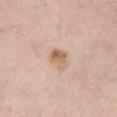Q: Was a biopsy performed?
A: imaged on a skin check; not biopsied
Q: Lesion location?
A: the left lower leg
Q: What kind of image is this?
A: total-body-photography crop, ~15 mm field of view
Q: What did automated image analysis measure?
A: a lesion color around L≈63 a*≈19 b*≈31 in CIELAB, roughly 11 lightness units darker than nearby skin, and a normalized border contrast of about 7.5; a lesion-detection confidence of about 100/100
Q: How large is the lesion?
A: about 2.5 mm
Q: How was the tile lit?
A: white-light illumination
Q: Patient demographics?
A: female, aged 43 to 47